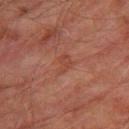  biopsy_status: not biopsied; imaged during a skin examination
  patient:
    sex: male
    age_approx: 75
  site: leg
  image:
    source: total-body photography crop
    field_of_view_mm: 15
  automated_metrics:
    border_irregularity_0_10: 4.0
    color_variation_0_10: 2.0
    peripheral_color_asymmetry: 1.0
  lighting: cross-polarized
  lesion_size:
    long_diameter_mm_approx: 2.5